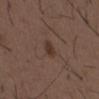Part of a total-body skin-imaging series; this lesion was reviewed on a skin check and was not flagged for biopsy.
A region of skin cropped from a whole-body photographic capture, roughly 15 mm wide.
Imaged with white-light lighting.
Located on the mid back.
The patient is a male aged 48 to 52.
Approximately 2.5 mm at its widest.